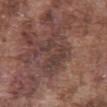Q: Is there a histopathology result?
A: imaged on a skin check; not biopsied
Q: Patient demographics?
A: male, approximately 75 years of age
Q: Automated lesion metrics?
A: an area of roughly 13 mm² and two-axis asymmetry of about 0.55; internal color variation of about 3.5 on a 0–10 scale and a peripheral color-asymmetry measure near 1
Q: How was the tile lit?
A: white-light
Q: How large is the lesion?
A: about 5.5 mm
Q: What is the anatomic site?
A: the abdomen
Q: What is the imaging modality?
A: ~15 mm tile from a whole-body skin photo